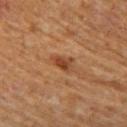Notes:
• workup · catalogued during a skin exam; not biopsied
• lighting · cross-polarized illumination
• acquisition · ~15 mm tile from a whole-body skin photo
• body site · the left thigh
• patient · male, approximately 65 years of age
• TBP lesion metrics · a lesion area of about 4.5 mm² and an outline eccentricity of about 0.8 (0 = round, 1 = elongated); an average lesion color of about L≈40 a*≈22 b*≈32 (CIELAB), about 9 CIELAB-L* units darker than the surrounding skin, and a normalized border contrast of about 7.5; a border-irregularity index near 3/10 and internal color variation of about 4 on a 0–10 scale
• lesion size · ≈3 mm